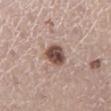{
  "biopsy_status": "not biopsied; imaged during a skin examination",
  "image": {
    "source": "total-body photography crop",
    "field_of_view_mm": 15
  },
  "patient": {
    "sex": "female",
    "age_approx": 45
  },
  "site": "leg"
}